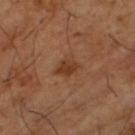Assessment:
The lesion was photographed on a routine skin check and not biopsied; there is no pathology result.
Background:
Measured at roughly 3 mm in maximum diameter. The lesion-visualizer software estimated a lesion area of about 4.5 mm², an eccentricity of roughly 0.7, and a symmetry-axis asymmetry near 0.2. A lesion tile, about 15 mm wide, cut from a 3D total-body photograph. Imaged with cross-polarized lighting. The lesion is on the left thigh. A male patient roughly 65 years of age.